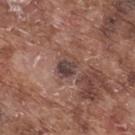Imaged with white-light lighting. A male patient, aged 73 to 77. An algorithmic analysis of the crop reported roughly 11 lightness units darker than nearby skin and a lesion-to-skin contrast of about 9.5 (normalized; higher = more distinct). It also reported an automated nevus-likeness rating near 20 out of 100 and a lesion-detection confidence of about 100/100. A roughly 15 mm field-of-view crop from a total-body skin photograph. The recorded lesion diameter is about 3 mm. The lesion is located on the upper back.